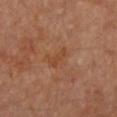<tbp_lesion>
<biopsy_status>not biopsied; imaged during a skin examination</biopsy_status>
<patient>
  <sex>female</sex>
  <age_approx>65</age_approx>
</patient>
<lighting>cross-polarized</lighting>
<image>
  <source>total-body photography crop</source>
  <field_of_view_mm>15</field_of_view_mm>
</image>
<site>right forearm</site>
<automated_metrics>
  <cielab_L>45</cielab_L>
  <cielab_a>24</cielab_a>
  <cielab_b>35</cielab_b>
  <vs_skin_contrast_norm>5.5</vs_skin_contrast_norm>
  <border_irregularity_0_10>4.0</border_irregularity_0_10>
  <nevus_likeness_0_100>0</nevus_likeness_0_100>
  <lesion_detection_confidence_0_100>100</lesion_detection_confidence_0_100>
</automated_metrics>
</tbp_lesion>